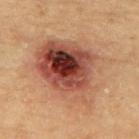workup: catalogued during a skin exam; not biopsied
acquisition: total-body-photography crop, ~15 mm field of view
subject: female, aged 58–62
anatomic site: the mid back
lighting: cross-polarized
lesion diameter: ≈9.5 mm